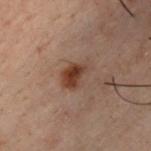Assessment:
Captured during whole-body skin photography for melanoma surveillance; the lesion was not biopsied.
Image and clinical context:
The lesion-visualizer software estimated a lesion-to-skin contrast of about 9 (normalized; higher = more distinct). The software also gave a within-lesion color-variation index near 4/10 and radial color variation of about 1. The software also gave a nevus-likeness score of about 95/100 and lesion-presence confidence of about 100/100. A roughly 15 mm field-of-view crop from a total-body skin photograph. Located on the chest. Captured under cross-polarized illumination. The patient is a male roughly 30 years of age. The lesion's longest dimension is about 4 mm.